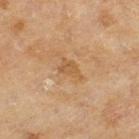The lesion was tiled from a total-body skin photograph and was not biopsied. A female subject in their 60s. On the left thigh. Captured under cross-polarized illumination. Measured at roughly 3 mm in maximum diameter. Cropped from a total-body skin-imaging series; the visible field is about 15 mm.